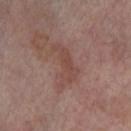anatomic site: the left thigh
subject: female, roughly 55 years of age
imaging modality: 15 mm crop, total-body photography